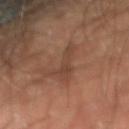Part of a total-body skin-imaging series; this lesion was reviewed on a skin check and was not flagged for biopsy. The lesion is located on the right upper arm. A male patient, aged 68–72. A 15 mm close-up tile from a total-body photography series done for melanoma screening.